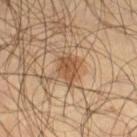Clinical impression: Recorded during total-body skin imaging; not selected for excision or biopsy. Clinical summary: A 15 mm close-up tile from a total-body photography series done for melanoma screening. An algorithmic analysis of the crop reported an area of roughly 7.5 mm², an eccentricity of roughly 0.6, and two-axis asymmetry of about 0.25. It also reported a border-irregularity index near 2.5/10, internal color variation of about 3 on a 0–10 scale, and peripheral color asymmetry of about 1. Located on the right thigh. The subject is a male aged 53 to 57.